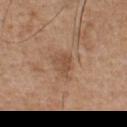notes = total-body-photography surveillance lesion; no biopsy
site = the chest
imaging modality = total-body-photography crop, ~15 mm field of view
size = about 2.5 mm
patient = male, aged around 55